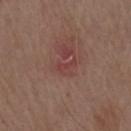  biopsy_status: not biopsied; imaged during a skin examination
  image:
    source: total-body photography crop
    field_of_view_mm: 15
  lighting: white-light
  patient:
    sex: male
    age_approx: 70
  site: right upper arm
  lesion_size:
    long_diameter_mm_approx: 2.5